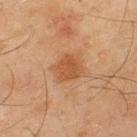Impression: The lesion was tiled from a total-body skin photograph and was not biopsied. Background: Captured under cross-polarized illumination. A 15 mm crop from a total-body photograph taken for skin-cancer surveillance. Measured at roughly 3.5 mm in maximum diameter. A male patient aged around 45. Located on the back.